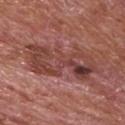This is a white-light tile.
About 9.5 mm across.
The patient is a male in their mid-60s.
Cropped from a total-body skin-imaging series; the visible field is about 15 mm.
Located on the chest.
Automated tile analysis of the lesion measured a lesion color around L≈43 a*≈25 b*≈24 in CIELAB, a lesion–skin lightness drop of about 9, and a normalized lesion–skin contrast near 7.5. And it measured a border-irregularity rating of about 7/10, a within-lesion color-variation index near 8.5/10, and radial color variation of about 3. And it measured an automated nevus-likeness rating near 0 out of 100 and a detector confidence of about 80 out of 100 that the crop contains a lesion.